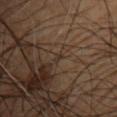Impression:
Recorded during total-body skin imaging; not selected for excision or biopsy.
Background:
On the upper back. A male subject, aged 58 to 62. Captured under cross-polarized illumination. A 15 mm crop from a total-body photograph taken for skin-cancer surveillance. Longest diameter approximately 1.5 mm. An algorithmic analysis of the crop reported a lesion color around L≈30 a*≈13 b*≈21 in CIELAB, roughly 5 lightness units darker than nearby skin, and a normalized border contrast of about 5.5. The software also gave border irregularity of about 4.5 on a 0–10 scale and peripheral color asymmetry of about 0.